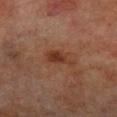Q: Was a biopsy performed?
A: no biopsy performed (imaged during a skin exam)
Q: What kind of image is this?
A: ~15 mm crop, total-body skin-cancer survey
Q: Automated lesion metrics?
A: an area of roughly 5.5 mm², an outline eccentricity of about 0.85 (0 = round, 1 = elongated), and a symmetry-axis asymmetry near 0.4; a border-irregularity index near 4/10, internal color variation of about 2 on a 0–10 scale, and a peripheral color-asymmetry measure near 0.5
Q: What is the anatomic site?
A: the leg
Q: Who is the patient?
A: male, aged around 70